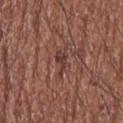Recorded during total-body skin imaging; not selected for excision or biopsy.
The lesion is located on the chest.
A 15 mm crop from a total-body photograph taken for skin-cancer surveillance.
A male subject, aged around 75.
Approximately 3 mm at its widest.